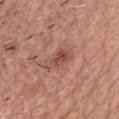<record>
<biopsy_status>not biopsied; imaged during a skin examination</biopsy_status>
<image>
  <source>total-body photography crop</source>
  <field_of_view_mm>15</field_of_view_mm>
</image>
<lesion_size>
  <long_diameter_mm_approx>4.0</long_diameter_mm_approx>
</lesion_size>
<site>front of the torso</site>
<patient>
  <sex>male</sex>
  <age_approx>55</age_approx>
</patient>
</record>